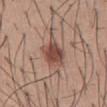Part of a total-body skin-imaging series; this lesion was reviewed on a skin check and was not flagged for biopsy.
An algorithmic analysis of the crop reported a footprint of about 9.5 mm², an outline eccentricity of about 0.75 (0 = round, 1 = elongated), and a symmetry-axis asymmetry near 0.25. The software also gave border irregularity of about 3 on a 0–10 scale and internal color variation of about 4.5 on a 0–10 scale. The software also gave a nevus-likeness score of about 100/100.
Imaged with white-light lighting.
From the abdomen.
Measured at roughly 4.5 mm in maximum diameter.
A male subject, aged around 30.
A region of skin cropped from a whole-body photographic capture, roughly 15 mm wide.